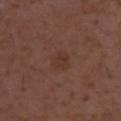Context: A male subject, roughly 50 years of age. The recorded lesion diameter is about 2.5 mm. A roughly 15 mm field-of-view crop from a total-body skin photograph. Imaged with white-light lighting.